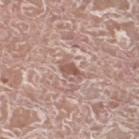Impression: The lesion was tiled from a total-body skin photograph and was not biopsied. Context: The patient is a male roughly 75 years of age. Captured under white-light illumination. Automated image analysis of the tile measured an area of roughly 3 mm², a shape eccentricity near 0.85, and a symmetry-axis asymmetry near 0.4. The analysis additionally found a mean CIELAB color near L≈55 a*≈20 b*≈24 and a lesion–skin lightness drop of about 11. The software also gave a nevus-likeness score of about 0/100 and a detector confidence of about 65 out of 100 that the crop contains a lesion. The recorded lesion diameter is about 3 mm. A 15 mm close-up tile from a total-body photography series done for melanoma screening. The lesion is located on the left lower leg.